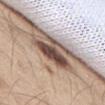Image and clinical context:
This image is a 15 mm lesion crop taken from a total-body photograph. A male patient, aged 58 to 62. Located on the right thigh.